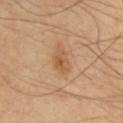Background:
A close-up tile cropped from a whole-body skin photograph, about 15 mm across. Approximately 3 mm at its widest. The patient is a male aged 38–42. Imaged with cross-polarized lighting. The total-body-photography lesion software estimated a border-irregularity rating of about 3/10, a color-variation rating of about 4/10, and a peripheral color-asymmetry measure near 1.5. The software also gave an automated nevus-likeness rating near 70 out of 100 and lesion-presence confidence of about 100/100. The lesion is located on the chest.